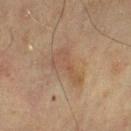Measured at roughly 4.5 mm in maximum diameter. A male subject aged 68 to 72. This is a cross-polarized tile. From the chest. A roughly 15 mm field-of-view crop from a total-body skin photograph.